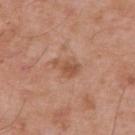workup: imaged on a skin check; not biopsied | lighting: white-light illumination | size: about 3.5 mm | image: 15 mm crop, total-body photography | location: the upper back | subject: male, approximately 55 years of age.